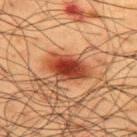Recorded during total-body skin imaging; not selected for excision or biopsy. The lesion is located on the upper back. The subject is a male aged around 60. The total-body-photography lesion software estimated a border-irregularity rating of about 2/10 and internal color variation of about 6.5 on a 0–10 scale. The recorded lesion diameter is about 5 mm. Cropped from a whole-body photographic skin survey; the tile spans about 15 mm.